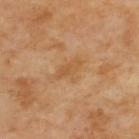The lesion was tiled from a total-body skin photograph and was not biopsied. Captured under cross-polarized illumination. The lesion's longest dimension is about 3.5 mm. Located on the upper back. A lesion tile, about 15 mm wide, cut from a 3D total-body photograph.